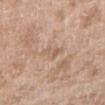site: right upper arm
image:
  source: total-body photography crop
  field_of_view_mm: 15
patient:
  sex: male
  age_approx: 50